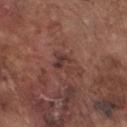Impression: Imaged during a routine full-body skin examination; the lesion was not biopsied and no histopathology is available. Background: Automated tile analysis of the lesion measured a lesion color around L≈37 a*≈20 b*≈21 in CIELAB and a normalized lesion–skin contrast near 6.5. The software also gave a border-irregularity index near 4.5/10 and a peripheral color-asymmetry measure near 0.5. This is a white-light tile. A close-up tile cropped from a whole-body skin photograph, about 15 mm across. A male subject, aged approximately 75. The lesion is located on the chest.